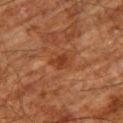biopsy_status: not biopsied; imaged during a skin examination
image:
  source: total-body photography crop
  field_of_view_mm: 15
automated_metrics:
  border_irregularity_0_10: 2.5
  color_variation_0_10: 2.0
  nevus_likeness_0_100: 0
  lesion_detection_confidence_0_100: 100
lesion_size:
  long_diameter_mm_approx: 3.0
site: right thigh
patient:
  sex: male
  age_approx: 80
lighting: cross-polarized